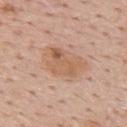The subject is a male aged 53 to 57. The lesion is located on the upper back. This is a white-light tile. A lesion tile, about 15 mm wide, cut from a 3D total-body photograph. The lesion-visualizer software estimated a footprint of about 12 mm² and a shape eccentricity near 0.75.